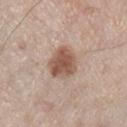Impression:
Imaged during a routine full-body skin examination; the lesion was not biopsied and no histopathology is available.
Image and clinical context:
From the right lower leg. A region of skin cropped from a whole-body photographic capture, roughly 15 mm wide. A male subject aged approximately 60.